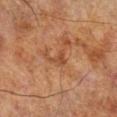Recorded during total-body skin imaging; not selected for excision or biopsy. Imaged with cross-polarized lighting. The lesion's longest dimension is about 2.5 mm. A male patient in their 70s. Automated image analysis of the tile measured a lesion color around L≈38 a*≈21 b*≈30 in CIELAB, about 7 CIELAB-L* units darker than the surrounding skin, and a lesion-to-skin contrast of about 6 (normalized; higher = more distinct). Located on the right lower leg. A region of skin cropped from a whole-body photographic capture, roughly 15 mm wide.